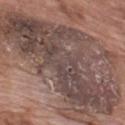The lesion was tiled from a total-body skin photograph and was not biopsied.
A male subject, approximately 70 years of age.
Approximately 17 mm at its widest.
A region of skin cropped from a whole-body photographic capture, roughly 15 mm wide.
The lesion-visualizer software estimated an eccentricity of roughly 0.85 and a shape-asymmetry score of about 0.45 (0 = symmetric). And it measured a color-variation rating of about 8/10 and radial color variation of about 2.5. It also reported a nevus-likeness score of about 0/100 and lesion-presence confidence of about 55/100.
From the upper back.